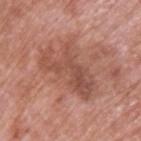Clinical impression: Recorded during total-body skin imaging; not selected for excision or biopsy. Clinical summary: Cropped from a total-body skin-imaging series; the visible field is about 15 mm. The tile uses white-light illumination. The lesion is located on the upper back. The lesion-visualizer software estimated an area of roughly 18 mm², an outline eccentricity of about 0.9 (0 = round, 1 = elongated), and two-axis asymmetry of about 0.5. The software also gave a mean CIELAB color near L≈51 a*≈24 b*≈28, roughly 9 lightness units darker than nearby skin, and a lesion-to-skin contrast of about 6.5 (normalized; higher = more distinct). A male patient, about 70 years old. Measured at roughly 7.5 mm in maximum diameter.